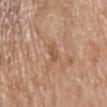workup=imaged on a skin check; not biopsied
diameter=about 2.5 mm
subject=male, aged 78–82
image source=15 mm crop, total-body photography
location=the chest